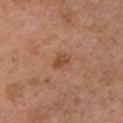{
  "biopsy_status": "not biopsied; imaged during a skin examination"
}